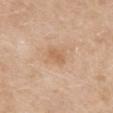Impression:
Imaged during a routine full-body skin examination; the lesion was not biopsied and no histopathology is available.
Acquisition and patient details:
On the upper back. A female patient, roughly 60 years of age. A 15 mm close-up tile from a total-body photography series done for melanoma screening. The recorded lesion diameter is about 2.5 mm. The lesion-visualizer software estimated an outline eccentricity of about 0.8 (0 = round, 1 = elongated) and a shape-asymmetry score of about 0.25 (0 = symmetric). It also reported a lesion color around L≈62 a*≈20 b*≈35 in CIELAB.